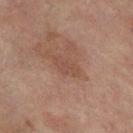biopsy status — catalogued during a skin exam; not biopsied
image — ~15 mm tile from a whole-body skin photo
lighting — cross-polarized illumination
automated metrics — a footprint of about 5.5 mm² and a shape-asymmetry score of about 0.25 (0 = symmetric); an average lesion color of about L≈43 a*≈18 b*≈25 (CIELAB); a border-irregularity index near 3/10, a within-lesion color-variation index near 2/10, and radial color variation of about 1
patient — female, about 80 years old
site — the right leg